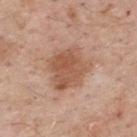Q: Was this lesion biopsied?
A: imaged on a skin check; not biopsied
Q: Who is the patient?
A: male, aged around 60
Q: What kind of image is this?
A: 15 mm crop, total-body photography
Q: Where on the body is the lesion?
A: the upper back
Q: Illumination type?
A: white-light
Q: What did automated image analysis measure?
A: an area of roughly 18 mm², a shape eccentricity near 0.5, and a shape-asymmetry score of about 0.15 (0 = symmetric); a classifier nevus-likeness of about 30/100
Q: Lesion size?
A: ≈5 mm